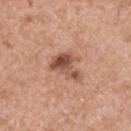This lesion was catalogued during total-body skin photography and was not selected for biopsy.
A 15 mm close-up extracted from a 3D total-body photography capture.
Located on the chest.
The subject is a male aged 68 to 72.